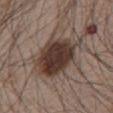A male patient in their mid- to late 60s. The recorded lesion diameter is about 7 mm. A 15 mm close-up extracted from a 3D total-body photography capture. From the chest. The total-body-photography lesion software estimated a classifier nevus-likeness of about 80/100 and a detector confidence of about 100 out of 100 that the crop contains a lesion.